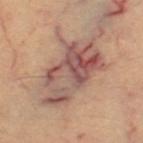No biopsy was performed on this lesion — it was imaged during a full skin examination and was not determined to be concerning. The patient is a female aged approximately 40. Imaged with cross-polarized lighting. An algorithmic analysis of the crop reported a footprint of about 31 mm², a shape eccentricity near 0.85, and two-axis asymmetry of about 0.3. The analysis additionally found a lesion color around L≈54 a*≈20 b*≈24 in CIELAB, a lesion–skin lightness drop of about 11, and a normalized lesion–skin contrast near 9. It also reported border irregularity of about 5.5 on a 0–10 scale, a color-variation rating of about 10/10, and radial color variation of about 4. On the left thigh. The lesion's longest dimension is about 9.5 mm. A lesion tile, about 15 mm wide, cut from a 3D total-body photograph.